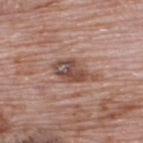Clinical impression:
The lesion was photographed on a routine skin check and not biopsied; there is no pathology result.
Acquisition and patient details:
The lesion is located on the upper back. A close-up tile cropped from a whole-body skin photograph, about 15 mm across. Automated image analysis of the tile measured a footprint of about 8.5 mm² and two-axis asymmetry of about 0.25. It also reported a border-irregularity index near 3/10, internal color variation of about 8.5 on a 0–10 scale, and peripheral color asymmetry of about 3. Captured under white-light illumination. Longest diameter approximately 5 mm. A male subject aged 68 to 72.